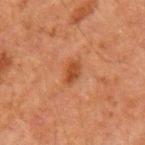workup — total-body-photography surveillance lesion; no biopsy
anatomic site — the left upper arm
size — ≈2.5 mm
lighting — cross-polarized illumination
subject — male, aged 58 to 62
image source — ~15 mm crop, total-body skin-cancer survey